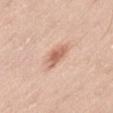- biopsy status · catalogued during a skin exam; not biopsied
- image source · 15 mm crop, total-body photography
- TBP lesion metrics · a mean CIELAB color near L≈64 a*≈22 b*≈30 and a lesion-to-skin contrast of about 7 (normalized; higher = more distinct); a border-irregularity index near 3/10, internal color variation of about 3.5 on a 0–10 scale, and a peripheral color-asymmetry measure near 1; a classifier nevus-likeness of about 75/100 and lesion-presence confidence of about 100/100
- patient · male, aged approximately 40
- body site · the left thigh
- lesion diameter · ≈4 mm
- lighting · white-light illumination